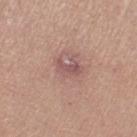follow-up: no biopsy performed (imaged during a skin exam) | subject: female, in their mid-50s | image-analysis metrics: a footprint of about 4 mm², a shape eccentricity near 0.8, and a symmetry-axis asymmetry near 0.5; an average lesion color of about L≈53 a*≈22 b*≈21 (CIELAB) and about 8 CIELAB-L* units darker than the surrounding skin; a nevus-likeness score of about 0/100 and a detector confidence of about 100 out of 100 that the crop contains a lesion | image: ~15 mm tile from a whole-body skin photo | anatomic site: the right thigh | lesion diameter: ~3 mm (longest diameter) | illumination: white-light illumination.